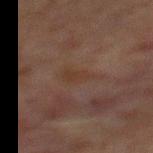image:
  source: total-body photography crop
  field_of_view_mm: 15
patient:
  sex: male
  age_approx: 65
site: chest
lighting: cross-polarized
lesion_size:
  long_diameter_mm_approx: 4.0